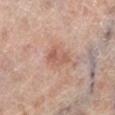Clinical impression:
Part of a total-body skin-imaging series; this lesion was reviewed on a skin check and was not flagged for biopsy.
Clinical summary:
The lesion-visualizer software estimated a nevus-likeness score of about 5/100. The tile uses white-light illumination. The lesion is located on the left lower leg. A female subject aged 63–67. Approximately 3 mm at its widest. A region of skin cropped from a whole-body photographic capture, roughly 15 mm wide.